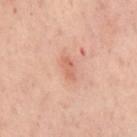Clinical summary: Located on the mid back. This is a cross-polarized tile. A lesion tile, about 15 mm wide, cut from a 3D total-body photograph. The lesion's longest dimension is about 3 mm. The patient is a female approximately 55 years of age. Automated image analysis of the tile measured an area of roughly 3 mm² and an outline eccentricity of about 0.9 (0 = round, 1 = elongated). And it measured a mean CIELAB color near L≈52 a*≈21 b*≈26 and a lesion-to-skin contrast of about 5.5 (normalized; higher = more distinct). The analysis additionally found a color-variation rating of about 1/10 and radial color variation of about 0.5.